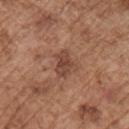biopsy status = total-body-photography surveillance lesion; no biopsy | patient = male, approximately 75 years of age | size = ≈3 mm | anatomic site = the chest | imaging modality = ~15 mm crop, total-body skin-cancer survey.